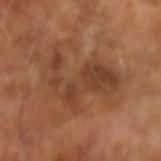Assessment:
Imaged during a routine full-body skin examination; the lesion was not biopsied and no histopathology is available.